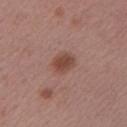Q: Was a biopsy performed?
A: imaged on a skin check; not biopsied
Q: What is the imaging modality?
A: ~15 mm crop, total-body skin-cancer survey
Q: How large is the lesion?
A: ~3 mm (longest diameter)
Q: What did automated image analysis measure?
A: an area of roughly 6 mm², a shape eccentricity near 0.55, and a symmetry-axis asymmetry near 0.2
Q: Who is the patient?
A: female, approximately 50 years of age
Q: Where on the body is the lesion?
A: the left upper arm